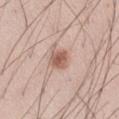Q: What did automated image analysis measure?
A: an area of roughly 5.5 mm², an eccentricity of roughly 0.65, and a symmetry-axis asymmetry near 0.2; a border-irregularity rating of about 2/10 and a within-lesion color-variation index near 3/10
Q: How was this image acquired?
A: ~15 mm crop, total-body skin-cancer survey
Q: Patient demographics?
A: male, approximately 30 years of age
Q: How was the tile lit?
A: white-light illumination
Q: How large is the lesion?
A: ≈3.5 mm
Q: Where on the body is the lesion?
A: the right thigh